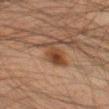Clinical impression: Captured during whole-body skin photography for melanoma surveillance; the lesion was not biopsied. Image and clinical context: Automated tile analysis of the lesion measured an area of roughly 7 mm² and a shape-asymmetry score of about 0.35 (0 = symmetric). And it measured about 9 CIELAB-L* units darker than the surrounding skin and a lesion-to-skin contrast of about 8.5 (normalized; higher = more distinct). And it measured a classifier nevus-likeness of about 95/100 and a detector confidence of about 100 out of 100 that the crop contains a lesion. A male subject, roughly 35 years of age. The lesion is located on the left thigh. A 15 mm close-up tile from a total-body photography series done for melanoma screening. Captured under cross-polarized illumination. Longest diameter approximately 4.5 mm.